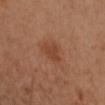Captured during whole-body skin photography for melanoma surveillance; the lesion was not biopsied. Automated tile analysis of the lesion measured a lesion-to-skin contrast of about 6 (normalized; higher = more distinct). And it measured internal color variation of about 1 on a 0–10 scale and radial color variation of about 0.5. The subject is a female about 40 years old. On the left arm. The recorded lesion diameter is about 3 mm. This is a cross-polarized tile. Cropped from a total-body skin-imaging series; the visible field is about 15 mm.